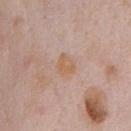| field | value |
|---|---|
| workup | no biopsy performed (imaged during a skin exam) |
| size | ≈2.5 mm |
| subject | male, aged approximately 60 |
| tile lighting | white-light |
| image source | 15 mm crop, total-body photography |
| location | the front of the torso |